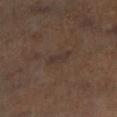Recorded during total-body skin imaging; not selected for excision or biopsy.
A male subject, aged around 60.
The total-body-photography lesion software estimated a border-irregularity rating of about 3.5/10 and a peripheral color-asymmetry measure near 0.5.
A roughly 15 mm field-of-view crop from a total-body skin photograph.
Longest diameter approximately 3 mm.
The lesion is located on the right lower leg.
Imaged with cross-polarized lighting.